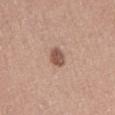<tbp_lesion>
  <biopsy_status>not biopsied; imaged during a skin examination</biopsy_status>
  <image>
    <source>total-body photography crop</source>
    <field_of_view_mm>15</field_of_view_mm>
  </image>
  <lesion_size>
    <long_diameter_mm_approx>2.5</long_diameter_mm_approx>
  </lesion_size>
  <automated_metrics>
    <vs_skin_contrast_norm>8.5</vs_skin_contrast_norm>
  </automated_metrics>
  <site>lower back</site>
  <patient>
    <sex>male</sex>
    <age_approx>40</age_approx>
  </patient>
  <lighting>white-light</lighting>
</tbp_lesion>